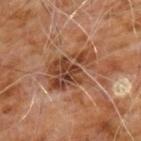follow-up=imaged on a skin check; not biopsied
automated lesion analysis=a mean CIELAB color near L≈42 a*≈22 b*≈31 and a lesion–skin lightness drop of about 11; a border-irregularity index near 5/10 and a color-variation rating of about 7/10
subject=male, in their 60s
diameter=≈6 mm
location=the chest
illumination=cross-polarized illumination
imaging modality=~15 mm crop, total-body skin-cancer survey